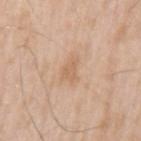Q: Was a biopsy performed?
A: imaged on a skin check; not biopsied
Q: What are the patient's age and sex?
A: male, in their mid-50s
Q: What is the imaging modality?
A: total-body-photography crop, ~15 mm field of view
Q: Lesion location?
A: the left upper arm